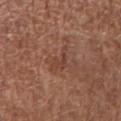Findings:
* follow-up · imaged on a skin check; not biopsied
* lesion size · ~3.5 mm (longest diameter)
* lighting · white-light illumination
* image-analysis metrics · a mean CIELAB color near L≈42 a*≈22 b*≈28, about 6 CIELAB-L* units darker than the surrounding skin, and a normalized border contrast of about 5.5
* imaging modality · ~15 mm tile from a whole-body skin photo
* site · the right upper arm
* subject · female, about 50 years old